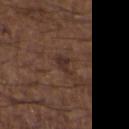biopsy status: no biopsy performed (imaged during a skin exam) | diameter: ≈3 mm | lighting: white-light | acquisition: 15 mm crop, total-body photography | site: the upper back | TBP lesion metrics: a lesion area of about 3.5 mm², a shape eccentricity near 0.85, and a shape-asymmetry score of about 0.4 (0 = symmetric); a mean CIELAB color near L≈30 a*≈17 b*≈21 and a normalized lesion–skin contrast near 7.5; an automated nevus-likeness rating near 0 out of 100 and a detector confidence of about 95 out of 100 that the crop contains a lesion | patient: male, about 50 years old.